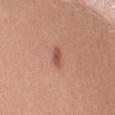workup: total-body-photography surveillance lesion; no biopsy
location: the abdomen
lighting: white-light
image source: total-body-photography crop, ~15 mm field of view
subject: female, aged around 35